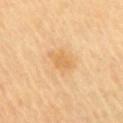Acquisition and patient details: The lesion's longest dimension is about 3 mm. Cropped from a total-body skin-imaging series; the visible field is about 15 mm. The subject is a female approximately 70 years of age. The tile uses cross-polarized illumination. On the front of the torso.